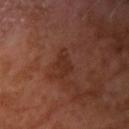| field | value |
|---|---|
| follow-up | imaged on a skin check; not biopsied |
| acquisition | 15 mm crop, total-body photography |
| patient | male, approximately 55 years of age |
| image-analysis metrics | a lesion color around L≈29 a*≈22 b*≈27 in CIELAB, roughly 5 lightness units darker than nearby skin, and a normalized border contrast of about 5.5; a border-irregularity rating of about 4.5/10 and internal color variation of about 1 on a 0–10 scale |
| location | the right upper arm |
| diameter | about 3.5 mm |